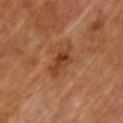The lesion was tiled from a total-body skin photograph and was not biopsied.
Automated image analysis of the tile measured a footprint of about 7 mm², a shape eccentricity near 0.9, and two-axis asymmetry of about 0.3. The software also gave an average lesion color of about L≈40 a*≈24 b*≈34 (CIELAB), about 9 CIELAB-L* units darker than the surrounding skin, and a normalized border contrast of about 7.5. The software also gave a border-irregularity rating of about 4/10 and internal color variation of about 4.5 on a 0–10 scale.
Captured under cross-polarized illumination.
Cropped from a whole-body photographic skin survey; the tile spans about 15 mm.
The lesion's longest dimension is about 5 mm.
A male subject about 65 years old.
The lesion is located on the chest.